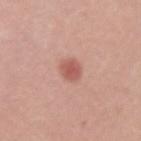Assessment: Recorded during total-body skin imaging; not selected for excision or biopsy. Image and clinical context: The tile uses white-light illumination. A female subject roughly 35 years of age. A lesion tile, about 15 mm wide, cut from a 3D total-body photograph. Longest diameter approximately 2.5 mm. From the left upper arm.